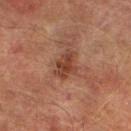notes — total-body-photography surveillance lesion; no biopsy
acquisition — total-body-photography crop, ~15 mm field of view
location — the left lower leg
automated metrics — a shape eccentricity near 0.75 and a symmetry-axis asymmetry near 0.25; a lesion color around L≈33 a*≈21 b*≈25 in CIELAB and a normalized border contrast of about 8; a within-lesion color-variation index near 3/10 and a peripheral color-asymmetry measure near 1; an automated nevus-likeness rating near 15 out of 100 and lesion-presence confidence of about 100/100
illumination — cross-polarized
size — about 3.5 mm
subject — male, aged around 75